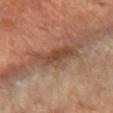Clinical impression: This lesion was catalogued during total-body skin photography and was not selected for biopsy. Clinical summary: Captured under cross-polarized illumination. On the left forearm. A female subject aged around 60. Cropped from a whole-body photographic skin survey; the tile spans about 15 mm.